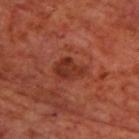Clinical impression:
Recorded during total-body skin imaging; not selected for excision or biopsy.
Background:
A male subject aged 68 to 72. A 15 mm close-up extracted from a 3D total-body photography capture. On the upper back.